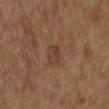Q: Was a biopsy performed?
A: imaged on a skin check; not biopsied
Q: What is the anatomic site?
A: the chest
Q: What is the imaging modality?
A: ~15 mm tile from a whole-body skin photo
Q: What are the patient's age and sex?
A: male, aged 73–77
Q: How large is the lesion?
A: about 3 mm
Q: Illumination type?
A: white-light illumination
Q: What did automated image analysis measure?
A: a footprint of about 4.5 mm², an eccentricity of roughly 0.7, and a shape-asymmetry score of about 0.2 (0 = symmetric); a lesion color around L≈40 a*≈18 b*≈27 in CIELAB, about 7 CIELAB-L* units darker than the surrounding skin, and a normalized lesion–skin contrast near 6; a color-variation rating of about 3/10 and peripheral color asymmetry of about 1; a nevus-likeness score of about 5/100 and a lesion-detection confidence of about 100/100